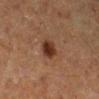The lesion was tiled from a total-body skin photograph and was not biopsied.
From the right lower leg.
The subject is a female aged 38 to 42.
Measured at roughly 2.5 mm in maximum diameter.
The tile uses cross-polarized illumination.
A 15 mm close-up tile from a total-body photography series done for melanoma screening.
An algorithmic analysis of the crop reported an area of roughly 5 mm². The analysis additionally found a border-irregularity rating of about 1.5/10, internal color variation of about 3 on a 0–10 scale, and a peripheral color-asymmetry measure near 1. It also reported a detector confidence of about 100 out of 100 that the crop contains a lesion.